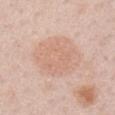{"biopsy_status": "not biopsied; imaged during a skin examination", "patient": {"sex": "male", "age_approx": 60}, "site": "right upper arm", "image": {"source": "total-body photography crop", "field_of_view_mm": 15}}